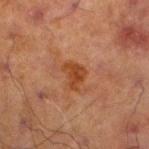Q: Was a biopsy performed?
A: no biopsy performed (imaged during a skin exam)
Q: What lighting was used for the tile?
A: cross-polarized illumination
Q: Patient demographics?
A: male, aged 68–72
Q: Lesion location?
A: the left lower leg
Q: How was this image acquired?
A: 15 mm crop, total-body photography